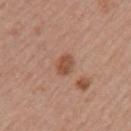Q: Was a biopsy performed?
A: catalogued during a skin exam; not biopsied
Q: What lighting was used for the tile?
A: white-light illumination
Q: What kind of image is this?
A: total-body-photography crop, ~15 mm field of view
Q: Automated lesion metrics?
A: an outline eccentricity of about 0.7 (0 = round, 1 = elongated) and a symmetry-axis asymmetry near 0.2; a within-lesion color-variation index near 3/10 and a peripheral color-asymmetry measure near 1; a nevus-likeness score of about 45/100 and lesion-presence confidence of about 100/100
Q: Who is the patient?
A: female, in their mid- to late 50s
Q: Lesion location?
A: the left upper arm
Q: How large is the lesion?
A: about 3 mm